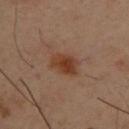Longest diameter approximately 3.5 mm. A male patient aged around 40. The tile uses cross-polarized illumination. The lesion is located on the back. A 15 mm crop from a total-body photograph taken for skin-cancer surveillance.